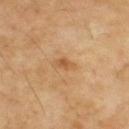No biopsy was performed on this lesion — it was imaged during a full skin examination and was not determined to be concerning. The lesion is located on the upper back. A region of skin cropped from a whole-body photographic capture, roughly 15 mm wide. The total-body-photography lesion software estimated a lesion area of about 2.5 mm² and an outline eccentricity of about 0.85 (0 = round, 1 = elongated). The lesion's longest dimension is about 2.5 mm. The patient is a male roughly 55 years of age. Captured under cross-polarized illumination.